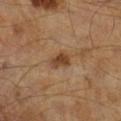| feature | finding |
|---|---|
| biopsy status | total-body-photography surveillance lesion; no biopsy |
| automated metrics | an area of roughly 4.5 mm², an eccentricity of roughly 0.75, and a shape-asymmetry score of about 0.25 (0 = symmetric); a lesion color around L≈42 a*≈19 b*≈32 in CIELAB and a lesion–skin lightness drop of about 10; a border-irregularity index near 2/10 and peripheral color asymmetry of about 1; a lesion-detection confidence of about 100/100 |
| diameter | ~3 mm (longest diameter) |
| image | ~15 mm crop, total-body skin-cancer survey |
| lighting | cross-polarized |
| patient | male, aged around 65 |